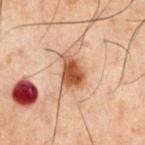<case>
  <biopsy_status>not biopsied; imaged during a skin examination</biopsy_status>
  <site>chest</site>
  <patient>
    <sex>male</sex>
    <age_approx>60</age_approx>
  </patient>
  <image>
    <source>total-body photography crop</source>
    <field_of_view_mm>15</field_of_view_mm>
  </image>
</case>